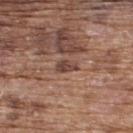{"biopsy_status": "not biopsied; imaged during a skin examination", "patient": {"sex": "male", "age_approx": 75}, "image": {"source": "total-body photography crop", "field_of_view_mm": 15}, "lesion_size": {"long_diameter_mm_approx": 2.5}, "site": "upper back"}